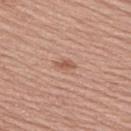notes: imaged on a skin check; not biopsied
automated lesion analysis: a footprint of about 2.5 mm², an outline eccentricity of about 0.85 (0 = round, 1 = elongated), and a symmetry-axis asymmetry near 0.25; a border-irregularity index near 2.5/10 and a peripheral color-asymmetry measure near 0; lesion-presence confidence of about 100/100
image: ~15 mm crop, total-body skin-cancer survey
illumination: white-light
patient: male, roughly 55 years of age
site: the upper back